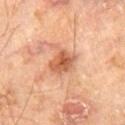The lesion was tiled from a total-body skin photograph and was not biopsied. From the right thigh. Automated image analysis of the tile measured an area of roughly 6.5 mm² and two-axis asymmetry of about 0.2. A male subject aged approximately 70. A 15 mm close-up tile from a total-body photography series done for melanoma screening. About 3 mm across. Captured under cross-polarized illumination.